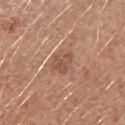The lesion is on the left forearm.
A 15 mm crop from a total-body photograph taken for skin-cancer surveillance.
A female patient aged around 40.